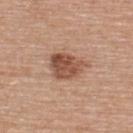Captured during whole-body skin photography for melanoma surveillance; the lesion was not biopsied. Approximately 4 mm at its widest. The patient is a male roughly 55 years of age. Captured under white-light illumination. A 15 mm crop from a total-body photograph taken for skin-cancer surveillance. Located on the upper back. Automated image analysis of the tile measured a mean CIELAB color near L≈51 a*≈22 b*≈30. The software also gave border irregularity of about 2.5 on a 0–10 scale, internal color variation of about 5 on a 0–10 scale, and a peripheral color-asymmetry measure near 2. And it measured a nevus-likeness score of about 65/100 and lesion-presence confidence of about 100/100.